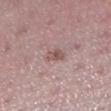notes = no biopsy performed (imaged during a skin exam); lighting = white-light illumination; patient = female, aged 43 to 47; diameter = about 2.5 mm; location = the leg; image source = 15 mm crop, total-body photography.